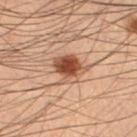follow-up = imaged on a skin check; not biopsied
location = the left lower leg
illumination = cross-polarized
size = ~4 mm (longest diameter)
acquisition = ~15 mm tile from a whole-body skin photo
patient = male, in their mid- to late 50s